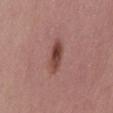| key | value |
|---|---|
| biopsy status | catalogued during a skin exam; not biopsied |
| tile lighting | white-light illumination |
| automated lesion analysis | internal color variation of about 5.5 on a 0–10 scale and a peripheral color-asymmetry measure near 2; an automated nevus-likeness rating near 95 out of 100 and lesion-presence confidence of about 100/100 |
| subject | male, aged approximately 55 |
| diameter | ≈4 mm |
| location | the mid back |
| image source | 15 mm crop, total-body photography |